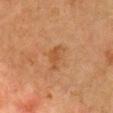  biopsy_status: not biopsied; imaged during a skin examination
  patient:
    sex: female
    age_approx: 50
  site: chest
  lesion_size:
    long_diameter_mm_approx: 3.5
  lighting: cross-polarized
  image:
    source: total-body photography crop
    field_of_view_mm: 15